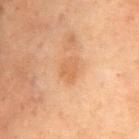Impression: Part of a total-body skin-imaging series; this lesion was reviewed on a skin check and was not flagged for biopsy.